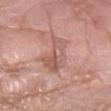<tbp_lesion>
<biopsy_status>not biopsied; imaged during a skin examination</biopsy_status>
<patient>
  <sex>male</sex>
  <age_approx>60</age_approx>
</patient>
<lighting>white-light</lighting>
<lesion_size>
  <long_diameter_mm_approx>5.0</long_diameter_mm_approx>
</lesion_size>
<site>right forearm</site>
<image>
  <source>total-body photography crop</source>
  <field_of_view_mm>15</field_of_view_mm>
</image>
</tbp_lesion>